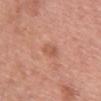Part of a total-body skin-imaging series; this lesion was reviewed on a skin check and was not flagged for biopsy.
The total-body-photography lesion software estimated roughly 8 lightness units darker than nearby skin and a normalized lesion–skin contrast near 5.5.
The subject is a female approximately 60 years of age.
A 15 mm close-up extracted from a 3D total-body photography capture.
On the chest.
Approximately 3 mm at its widest.